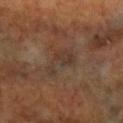Background: From the right forearm. The subject is a female aged around 60. Cropped from a whole-body photographic skin survey; the tile spans about 15 mm. The tile uses cross-polarized illumination. The lesion's longest dimension is about 4 mm.